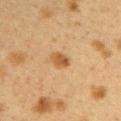<case>
<lesion_size>
  <long_diameter_mm_approx>3.0</long_diameter_mm_approx>
</lesion_size>
<site>back</site>
<image>
  <source>total-body photography crop</source>
  <field_of_view_mm>15</field_of_view_mm>
</image>
<patient>
  <sex>female</sex>
  <age_approx>40</age_approx>
</patient>
</case>